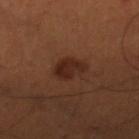* notes: imaged on a skin check; not biopsied
* image: ~15 mm tile from a whole-body skin photo
* body site: the right lower leg
* subject: male, aged around 50
* lesion diameter: about 3.5 mm
* automated metrics: a lesion–skin lightness drop of about 8 and a normalized lesion–skin contrast near 8.5; a border-irregularity index near 2/10 and a color-variation rating of about 2.5/10; an automated nevus-likeness rating near 90 out of 100 and a detector confidence of about 100 out of 100 that the crop contains a lesion